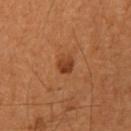Clinical impression:
The lesion was tiled from a total-body skin photograph and was not biopsied.
Image and clinical context:
A lesion tile, about 15 mm wide, cut from a 3D total-body photograph. A female patient, aged approximately 50. On the right upper arm.